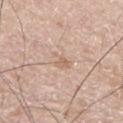Q: Is there a histopathology result?
A: total-body-photography surveillance lesion; no biopsy
Q: What is the anatomic site?
A: the right upper arm
Q: What is the imaging modality?
A: ~15 mm tile from a whole-body skin photo
Q: Patient demographics?
A: male, in their mid-70s